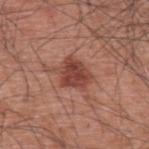site=the upper back; image source=~15 mm tile from a whole-body skin photo; size=about 3.5 mm; illumination=white-light; automated lesion analysis=a lesion area of about 9.5 mm², an eccentricity of roughly 0.55, and a symmetry-axis asymmetry near 0.25; patient=male, aged 58 to 62.